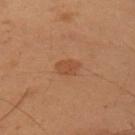notes: catalogued during a skin exam; not biopsied
lesion diameter: ~2.5 mm (longest diameter)
image: total-body-photography crop, ~15 mm field of view
lighting: cross-polarized
site: the right upper arm
subject: male, approximately 55 years of age